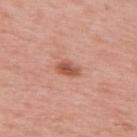{
  "biopsy_status": "not biopsied; imaged during a skin examination",
  "lesion_size": {
    "long_diameter_mm_approx": 2.5
  },
  "patient": {
    "sex": "female",
    "age_approx": 60
  },
  "site": "back",
  "image": {
    "source": "total-body photography crop",
    "field_of_view_mm": 15
  }
}